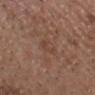workup = imaged on a skin check; not biopsied | body site = the head or neck | lighting = white-light | size = about 2.5 mm | image = total-body-photography crop, ~15 mm field of view | patient = male, aged approximately 55.